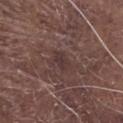The total-body-photography lesion software estimated an area of roughly 4 mm² and a shape-asymmetry score of about 0.25 (0 = symmetric). It also reported a nevus-likeness score of about 0/100 and lesion-presence confidence of about 70/100. The lesion is located on the front of the torso. Cropped from a total-body skin-imaging series; the visible field is about 15 mm. The tile uses white-light illumination. About 3 mm across. The subject is a male aged approximately 80.